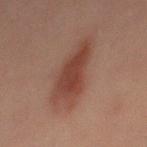This lesion was catalogued during total-body skin photography and was not selected for biopsy.